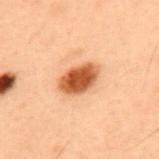Case summary:
• workup — total-body-photography surveillance lesion; no biopsy
• acquisition — ~15 mm tile from a whole-body skin photo
• site — the back
• lighting — cross-polarized illumination
• lesion diameter — about 4.5 mm
• patient — male, about 55 years old